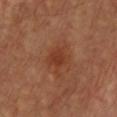Assessment: Captured during whole-body skin photography for melanoma surveillance; the lesion was not biopsied. Clinical summary: The tile uses cross-polarized illumination. A lesion tile, about 15 mm wide, cut from a 3D total-body photograph. A male subject about 40 years old. The recorded lesion diameter is about 3 mm. On the front of the torso.